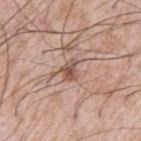No biopsy was performed on this lesion — it was imaged during a full skin examination and was not determined to be concerning.
The patient is a male in their 60s.
The tile uses white-light illumination.
The recorded lesion diameter is about 2.5 mm.
Located on the left thigh.
A region of skin cropped from a whole-body photographic capture, roughly 15 mm wide.